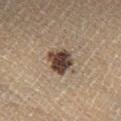  biopsy_status: not biopsied; imaged during a skin examination
  image:
    source: total-body photography crop
    field_of_view_mm: 15
  site: right lower leg
  lighting: cross-polarized
  automated_metrics:
    area_mm2_approx: 8.0
    shape_asymmetry: 0.25
    cielab_L: 32
    cielab_a: 12
    cielab_b: 20
    vs_skin_darker_L: 15.0
    vs_skin_contrast_norm: 13.5
    color_variation_0_10: 4.5
    peripheral_color_asymmetry: 1.0
    nevus_likeness_0_100: 85
    lesion_detection_confidence_0_100: 100
  lesion_size:
    long_diameter_mm_approx: 3.5
  patient:
    sex: male
    age_approx: 65